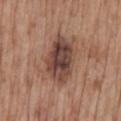Context:
The lesion's longest dimension is about 6 mm. The total-body-photography lesion software estimated a color-variation rating of about 7/10 and radial color variation of about 2.5. From the back. The patient is a male roughly 70 years of age. A 15 mm close-up extracted from a 3D total-body photography capture. This is a white-light tile.